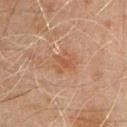Assessment:
This lesion was catalogued during total-body skin photography and was not selected for biopsy.
Image and clinical context:
The recorded lesion diameter is about 2.5 mm. This is a cross-polarized tile. Cropped from a total-body skin-imaging series; the visible field is about 15 mm. The total-body-photography lesion software estimated a border-irregularity rating of about 7/10, internal color variation of about 0.5 on a 0–10 scale, and radial color variation of about 0. A male patient, in their mid- to late 40s. The lesion is located on the chest.